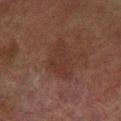Image and clinical context:
Imaged with cross-polarized lighting. A male subject aged around 75. The recorded lesion diameter is about 5 mm. Automated image analysis of the tile measured a border-irregularity rating of about 5.5/10 and a peripheral color-asymmetry measure near 0.5. And it measured a classifier nevus-likeness of about 5/100 and a detector confidence of about 100 out of 100 that the crop contains a lesion. A 15 mm close-up tile from a total-body photography series done for melanoma screening. The lesion is located on the arm.